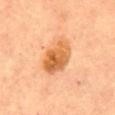Part of a total-body skin-imaging series; this lesion was reviewed on a skin check and was not flagged for biopsy.
Imaged with cross-polarized lighting.
This image is a 15 mm lesion crop taken from a total-body photograph.
The total-body-photography lesion software estimated an area of roughly 13 mm², a shape eccentricity near 0.75, and a shape-asymmetry score of about 0.2 (0 = symmetric). And it measured a lesion color around L≈56 a*≈24 b*≈41 in CIELAB and a normalized lesion–skin contrast near 9. The analysis additionally found border irregularity of about 2 on a 0–10 scale, internal color variation of about 6.5 on a 0–10 scale, and peripheral color asymmetry of about 2.5.
Approximately 5 mm at its widest.
Located on the abdomen.
The subject is a female aged 58 to 62.